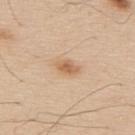Q: Is there a histopathology result?
A: no biopsy performed (imaged during a skin exam)